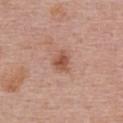Impression: Captured during whole-body skin photography for melanoma surveillance; the lesion was not biopsied. Acquisition and patient details: A region of skin cropped from a whole-body photographic capture, roughly 15 mm wide. On the upper back. The subject is a female approximately 65 years of age. Imaged with white-light lighting. The recorded lesion diameter is about 2.5 mm.